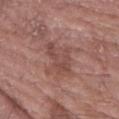lesion size: ~4.5 mm (longest diameter)
body site: the arm
imaging modality: total-body-photography crop, ~15 mm field of view
TBP lesion metrics: an area of roughly 9 mm², an outline eccentricity of about 0.8 (0 = round, 1 = elongated), and a symmetry-axis asymmetry near 0.3
subject: male, about 60 years old